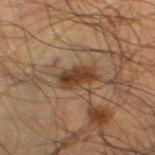The lesion was tiled from a total-body skin photograph and was not biopsied. A male subject aged 48–52. On the left thigh. A 15 mm close-up extracted from a 3D total-body photography capture.